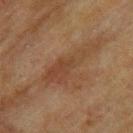{
  "site": "upper back",
  "automated_metrics": {
    "area_mm2_approx": 13.0,
    "shape_asymmetry": 0.45,
    "cielab_L": 35,
    "cielab_a": 16,
    "cielab_b": 27,
    "lesion_detection_confidence_0_100": 95
  },
  "image": {
    "source": "total-body photography crop",
    "field_of_view_mm": 15
  },
  "lighting": "cross-polarized",
  "patient": {
    "sex": "male",
    "age_approx": 75
  }
}